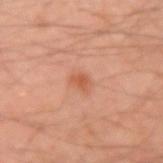Notes:
– workup: imaged on a skin check; not biopsied
– lesion diameter: ≈2 mm
– site: the right upper arm
– illumination: cross-polarized
– patient: male, in their mid- to late 40s
– image: ~15 mm tile from a whole-body skin photo